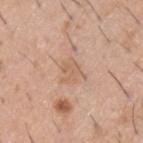The lesion was tiled from a total-body skin photograph and was not biopsied. The lesion is on the back. A male patient aged approximately 60. Automated tile analysis of the lesion measured about 7 CIELAB-L* units darker than the surrounding skin and a lesion-to-skin contrast of about 5 (normalized; higher = more distinct). About 3 mm across. This image is a 15 mm lesion crop taken from a total-body photograph.